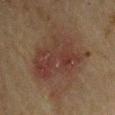body site: the right upper arm; patient: male, roughly 65 years of age; lesion size: ≈7.5 mm; illumination: cross-polarized illumination; image: total-body-photography crop, ~15 mm field of view.